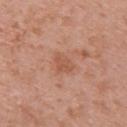This is a white-light tile.
A lesion tile, about 15 mm wide, cut from a 3D total-body photograph.
The subject is a female aged around 50.
The lesion is on the upper back.